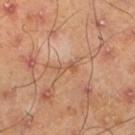Captured during whole-body skin photography for melanoma surveillance; the lesion was not biopsied. Captured under cross-polarized illumination. On the leg. A male subject about 45 years old. The lesion-visualizer software estimated a mean CIELAB color near L≈55 a*≈21 b*≈33 and a lesion-to-skin contrast of about 5 (normalized; higher = more distinct). It also reported a border-irregularity rating of about 7/10 and a color-variation rating of about 0/10. Longest diameter approximately 3.5 mm. A lesion tile, about 15 mm wide, cut from a 3D total-body photograph.